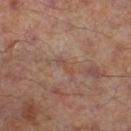Impression:
This lesion was catalogued during total-body skin photography and was not selected for biopsy.
Clinical summary:
A lesion tile, about 15 mm wide, cut from a 3D total-body photograph. From the right lower leg. A male patient roughly 65 years of age. The recorded lesion diameter is about 2.5 mm.